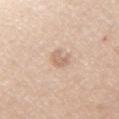Impression: Recorded during total-body skin imaging; not selected for excision or biopsy. Acquisition and patient details: Located on the right upper arm. Measured at roughly 3 mm in maximum diameter. A male patient in their 40s. This is a white-light tile. Cropped from a whole-body photographic skin survey; the tile spans about 15 mm.